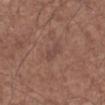This lesion was catalogued during total-body skin photography and was not selected for biopsy. The patient is a male aged 53–57. The lesion-visualizer software estimated a symmetry-axis asymmetry near 0.35. The software also gave a mean CIELAB color near L≈45 a*≈19 b*≈23, about 5 CIELAB-L* units darker than the surrounding skin, and a normalized lesion–skin contrast near 5. The analysis additionally found a border-irregularity rating of about 3.5/10, a within-lesion color-variation index near 0.5/10, and a peripheral color-asymmetry measure near 0. A 15 mm close-up extracted from a 3D total-body photography capture. This is a white-light tile. The lesion is located on the left forearm.